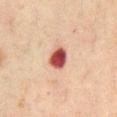Q: Is there a histopathology result?
A: no biopsy performed (imaged during a skin exam)
Q: Who is the patient?
A: male, approximately 70 years of age
Q: Lesion location?
A: the mid back
Q: What kind of image is this?
A: 15 mm crop, total-body photography